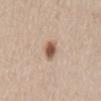diameter = ≈3 mm | automated lesion analysis = a border-irregularity index near 2.5/10, internal color variation of about 3.5 on a 0–10 scale, and peripheral color asymmetry of about 1 | patient = male, roughly 60 years of age | image source = total-body-photography crop, ~15 mm field of view | site = the front of the torso.